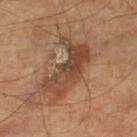Imaged during a routine full-body skin examination; the lesion was not biopsied and no histopathology is available. Captured under cross-polarized illumination. The recorded lesion diameter is about 7 mm. The subject is a male aged 63–67. A lesion tile, about 15 mm wide, cut from a 3D total-body photograph. On the left lower leg.